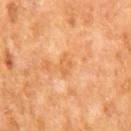biopsy status: catalogued during a skin exam; not biopsied
patient: male, roughly 65 years of age
tile lighting: cross-polarized illumination
lesion diameter: about 3 mm
anatomic site: the mid back
image: ~15 mm crop, total-body skin-cancer survey
image-analysis metrics: border irregularity of about 3 on a 0–10 scale and peripheral color asymmetry of about 0.5